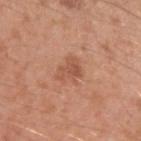Part of a total-body skin-imaging series; this lesion was reviewed on a skin check and was not flagged for biopsy.
The lesion is on the left upper arm.
Cropped from a whole-body photographic skin survey; the tile spans about 15 mm.
The total-body-photography lesion software estimated a footprint of about 6 mm², an outline eccentricity of about 0.7 (0 = round, 1 = elongated), and two-axis asymmetry of about 0.4. The analysis additionally found a lesion color around L≈54 a*≈24 b*≈32 in CIELAB and a normalized border contrast of about 5.5. The analysis additionally found a nevus-likeness score of about 5/100 and a detector confidence of about 100 out of 100 that the crop contains a lesion.
The subject is a male roughly 30 years of age.
The lesion's longest dimension is about 3.5 mm.